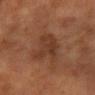* workup: no biopsy performed (imaged during a skin exam)
* subject: female, aged 48–52
* image: total-body-photography crop, ~15 mm field of view
* location: the arm